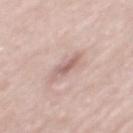Case summary:
* biopsy status · catalogued during a skin exam; not biopsied
* size · about 4 mm
* acquisition · total-body-photography crop, ~15 mm field of view
* automated metrics · a border-irregularity index near 4.5/10
* anatomic site · the mid back
* lighting · white-light illumination
* patient · male, approximately 55 years of age